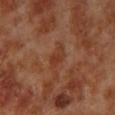follow-up: catalogued during a skin exam; not biopsied | body site: the left lower leg | TBP lesion metrics: a lesion color around L≈36 a*≈24 b*≈31 in CIELAB and a lesion-to-skin contrast of about 6 (normalized; higher = more distinct); an automated nevus-likeness rating near 0 out of 100 and a detector confidence of about 100 out of 100 that the crop contains a lesion | image source: ~15 mm tile from a whole-body skin photo | patient: male, aged 68 to 72.